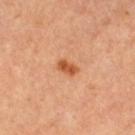Case summary:
• biopsy status — catalogued during a skin exam; not biopsied
• automated metrics — an area of roughly 3.5 mm² and a shape-asymmetry score of about 0.3 (0 = symmetric); a lesion color around L≈57 a*≈29 b*≈41 in CIELAB, roughly 12 lightness units darker than nearby skin, and a lesion-to-skin contrast of about 8.5 (normalized; higher = more distinct); an automated nevus-likeness rating near 95 out of 100 and lesion-presence confidence of about 100/100
• location — the right thigh
• patient — female, aged 28–32
• lighting — cross-polarized
• imaging modality — total-body-photography crop, ~15 mm field of view